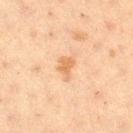<lesion>
  <lesion_size>
    <long_diameter_mm_approx>3.0</long_diameter_mm_approx>
  </lesion_size>
  <patient>
    <sex>female</sex>
    <age_approx>40</age_approx>
  </patient>
  <image>
    <source>total-body photography crop</source>
    <field_of_view_mm>15</field_of_view_mm>
  </image>
  <lighting>cross-polarized</lighting>
  <site>right thigh</site>
</lesion>